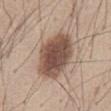biopsy status: imaged on a skin check; not biopsied | patient: male, aged 43–47 | acquisition: 15 mm crop, total-body photography | automated lesion analysis: a footprint of about 23 mm², an outline eccentricity of about 0.8 (0 = round, 1 = elongated), and a shape-asymmetry score of about 0.1 (0 = symmetric); about 16 CIELAB-L* units darker than the surrounding skin and a normalized border contrast of about 11; a border-irregularity rating of about 1.5/10 and peripheral color asymmetry of about 1.5; an automated nevus-likeness rating near 95 out of 100 and a lesion-detection confidence of about 100/100 | site: the abdomen.